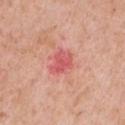notes = no biopsy performed (imaged during a skin exam)
acquisition = total-body-photography crop, ~15 mm field of view
lesion diameter = ~3 mm (longest diameter)
automated lesion analysis = a mean CIELAB color near L≈58 a*≈37 b*≈27 and a lesion–skin lightness drop of about 10; a classifier nevus-likeness of about 0/100 and a lesion-detection confidence of about 100/100
lighting = white-light
subject = female, aged 38–42
site = the chest